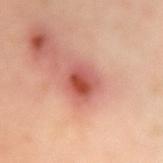Case summary:
- follow-up — total-body-photography surveillance lesion; no biopsy
- TBP lesion metrics — a lesion area of about 10 mm², an eccentricity of roughly 0.6, and two-axis asymmetry of about 0.15; an average lesion color of about L≈57 a*≈31 b*≈30 (CIELAB), roughly 13 lightness units darker than nearby skin, and a lesion-to-skin contrast of about 8.5 (normalized; higher = more distinct); border irregularity of about 1.5 on a 0–10 scale and a peripheral color-asymmetry measure near 2.5
- tile lighting — cross-polarized illumination
- subject — female, aged 38–42
- diameter — ≈4 mm
- imaging modality — total-body-photography crop, ~15 mm field of view
- location — the left arm